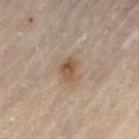Impression:
This lesion was catalogued during total-body skin photography and was not selected for biopsy.
Clinical summary:
The lesion is located on the left thigh. Longest diameter approximately 2.5 mm. A lesion tile, about 15 mm wide, cut from a 3D total-body photograph. A female subject roughly 60 years of age.